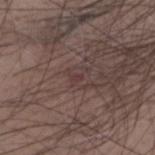Assessment:
This lesion was catalogued during total-body skin photography and was not selected for biopsy.
Image and clinical context:
Longest diameter approximately 1 mm. The tile uses white-light illumination. A male subject about 50 years old. On the head or neck. A region of skin cropped from a whole-body photographic capture, roughly 15 mm wide.